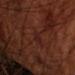Background:
The subject is a male aged 68–72. Automated image analysis of the tile measured a border-irregularity rating of about 3/10, a color-variation rating of about 1/10, and radial color variation of about 0.5. A roughly 15 mm field-of-view crop from a total-body skin photograph. Longest diameter approximately 3 mm. Imaged with cross-polarized lighting. On the arm.